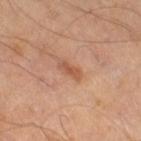{"biopsy_status": "not biopsied; imaged during a skin examination", "site": "leg", "lesion_size": {"long_diameter_mm_approx": 3.0}, "patient": {"sex": "male", "age_approx": 65}, "lighting": "cross-polarized", "image": {"source": "total-body photography crop", "field_of_view_mm": 15}}